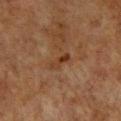workup: catalogued during a skin exam; not biopsied
acquisition: 15 mm crop, total-body photography
anatomic site: the chest
subject: female, aged approximately 55